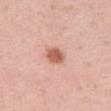biopsy status: total-body-photography surveillance lesion; no biopsy | size: ~2.5 mm (longest diameter) | image-analysis metrics: a footprint of about 4.5 mm², an eccentricity of roughly 0.55, and a symmetry-axis asymmetry near 0.25; an average lesion color of about L≈60 a*≈26 b*≈30 (CIELAB), a lesion–skin lightness drop of about 14, and a lesion-to-skin contrast of about 9 (normalized; higher = more distinct) | acquisition: total-body-photography crop, ~15 mm field of view | lighting: white-light | subject: female, approximately 45 years of age | site: the left thigh.